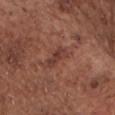biopsy_status: not biopsied; imaged during a skin examination
site: chest
patient:
  sex: male
  age_approx: 75
automated_metrics:
  color_variation_0_10: 2.0
  peripheral_color_asymmetry: 0.5
  nevus_likeness_0_100: 0
  lesion_detection_confidence_0_100: 100
image:
  source: total-body photography crop
  field_of_view_mm: 15
lesion_size:
  long_diameter_mm_approx: 3.0
lighting: white-light